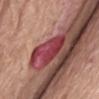workup — no biopsy performed (imaged during a skin exam); subject — female, about 75 years old; site — the right thigh; image source — total-body-photography crop, ~15 mm field of view.